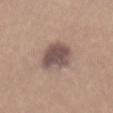Findings:
• follow-up · catalogued during a skin exam; not biopsied
• diameter · ≈5.5 mm
• body site · the abdomen
• patient · male, aged approximately 25
• TBP lesion metrics · a lesion color around L≈51 a*≈15 b*≈20 in CIELAB and about 13 CIELAB-L* units darker than the surrounding skin; internal color variation of about 4.5 on a 0–10 scale and radial color variation of about 1.5
• lighting · white-light illumination
• imaging modality · 15 mm crop, total-body photography